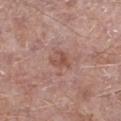No biopsy was performed on this lesion — it was imaged during a full skin examination and was not determined to be concerning.
A close-up tile cropped from a whole-body skin photograph, about 15 mm across.
A male patient, aged around 55.
The lesion's longest dimension is about 2.5 mm.
Imaged with white-light lighting.
The lesion is on the left lower leg.